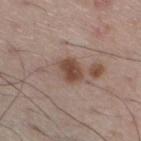  biopsy_status: not biopsied; imaged during a skin examination
  lighting: white-light
  lesion_size:
    long_diameter_mm_approx: 3.0
  site: left lower leg
  image:
    source: total-body photography crop
    field_of_view_mm: 15
  patient:
    sex: male
    age_approx: 60
  automated_metrics:
    border_irregularity_0_10: 2.0
    color_variation_0_10: 2.5